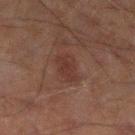Captured during whole-body skin photography for melanoma surveillance; the lesion was not biopsied. This image is a 15 mm lesion crop taken from a total-body photograph. The tile uses cross-polarized illumination. About 3.5 mm across. Located on the left lower leg. A male patient, approximately 60 years of age.